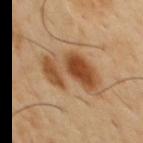follow-up: imaged on a skin check; not biopsied
location: the chest
imaging modality: ~15 mm tile from a whole-body skin photo
subject: male, about 50 years old